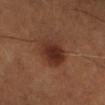{
  "biopsy_status": "not biopsied; imaged during a skin examination",
  "lesion_size": {
    "long_diameter_mm_approx": 4.5
  },
  "automated_metrics": {
    "eccentricity": 0.65,
    "shape_asymmetry": 0.15,
    "cielab_L": 31,
    "cielab_a": 21,
    "cielab_b": 26,
    "vs_skin_darker_L": 9.0,
    "vs_skin_contrast_norm": 9.0,
    "border_irregularity_0_10": 1.5,
    "color_variation_0_10": 4.0,
    "peripheral_color_asymmetry": 1.0,
    "nevus_likeness_0_100": 95,
    "lesion_detection_confidence_0_100": 100
  },
  "site": "right lower leg",
  "image": {
    "source": "total-body photography crop",
    "field_of_view_mm": 15
  },
  "patient": {
    "sex": "male",
    "age_approx": 50
  },
  "lighting": "cross-polarized"
}